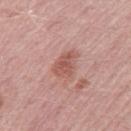notes = imaged on a skin check; not biopsied
patient = male, aged approximately 50
anatomic site = the right thigh
image = ~15 mm tile from a whole-body skin photo